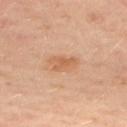Q: Is there a histopathology result?
A: catalogued during a skin exam; not biopsied
Q: Illumination type?
A: cross-polarized illumination
Q: Where on the body is the lesion?
A: the chest
Q: What are the patient's age and sex?
A: female, aged around 50
Q: Automated lesion metrics?
A: a footprint of about 6 mm², an outline eccentricity of about 0.85 (0 = round, 1 = elongated), and a symmetry-axis asymmetry near 0.3; an average lesion color of about L≈59 a*≈22 b*≈35 (CIELAB) and a normalized border contrast of about 6; a border-irregularity rating of about 3/10 and a peripheral color-asymmetry measure near 0.5; a detector confidence of about 100 out of 100 that the crop contains a lesion
Q: Lesion size?
A: ≈3.5 mm
Q: What is the imaging modality?
A: ~15 mm crop, total-body skin-cancer survey